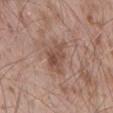| feature | finding |
|---|---|
| follow-up | total-body-photography surveillance lesion; no biopsy |
| tile lighting | white-light illumination |
| image source | total-body-photography crop, ~15 mm field of view |
| size | ~4.5 mm (longest diameter) |
| image-analysis metrics | a mean CIELAB color near L≈50 a*≈19 b*≈26 and a normalized lesion–skin contrast near 6.5; a nevus-likeness score of about 0/100 and a lesion-detection confidence of about 100/100 |
| patient | male, aged 53 to 57 |
| site | the lower back |